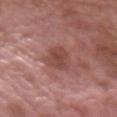Clinical impression: The lesion was tiled from a total-body skin photograph and was not biopsied. Acquisition and patient details: Captured under white-light illumination. From the left forearm. A lesion tile, about 15 mm wide, cut from a 3D total-body photograph. About 3.5 mm across. A male patient, approximately 40 years of age.